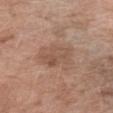Assessment: Part of a total-body skin-imaging series; this lesion was reviewed on a skin check and was not flagged for biopsy. Context: Longest diameter approximately 4 mm. Automated image analysis of the tile measured an average lesion color of about L≈52 a*≈19 b*≈28 (CIELAB) and roughly 7 lightness units darker than nearby skin. The software also gave a classifier nevus-likeness of about 0/100. A 15 mm close-up extracted from a 3D total-body photography capture. A female patient roughly 75 years of age. From the right forearm. Captured under white-light illumination.